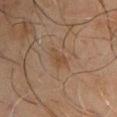The lesion was photographed on a routine skin check and not biopsied; there is no pathology result.